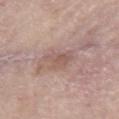Imaged during a routine full-body skin examination; the lesion was not biopsied and no histopathology is available. An algorithmic analysis of the crop reported border irregularity of about 4 on a 0–10 scale, internal color variation of about 3.5 on a 0–10 scale, and a peripheral color-asymmetry measure near 1. And it measured a classifier nevus-likeness of about 0/100 and a detector confidence of about 100 out of 100 that the crop contains a lesion. A female subject, roughly 70 years of age. Imaged with white-light lighting. About 4 mm across. Located on the left upper arm. A lesion tile, about 15 mm wide, cut from a 3D total-body photograph.